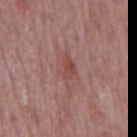This lesion was catalogued during total-body skin photography and was not selected for biopsy. On the mid back. The patient is a male in their mid-70s. An algorithmic analysis of the crop reported internal color variation of about 1.5 on a 0–10 scale and radial color variation of about 0.5. Cropped from a whole-body photographic skin survey; the tile spans about 15 mm. The lesion's longest dimension is about 2.5 mm. The tile uses white-light illumination.